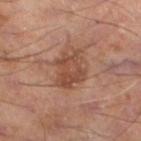Clinical impression:
Imaged during a routine full-body skin examination; the lesion was not biopsied and no histopathology is available.
Acquisition and patient details:
Located on the left lower leg. A 15 mm close-up tile from a total-body photography series done for melanoma screening. The tile uses cross-polarized illumination. A male subject aged 53 to 57. Automated image analysis of the tile measured an automated nevus-likeness rating near 40 out of 100 and a detector confidence of about 100 out of 100 that the crop contains a lesion. Measured at roughly 4 mm in maximum diameter.